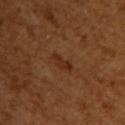Notes:
- tile lighting: cross-polarized
- anatomic site: the upper back
- diameter: about 3 mm
- image source: total-body-photography crop, ~15 mm field of view
- image-analysis metrics: an area of roughly 4 mm², a shape eccentricity near 0.9, and two-axis asymmetry of about 0.3; a border-irregularity index near 4/10, a within-lesion color-variation index near 1.5/10, and a peripheral color-asymmetry measure near 0.5; a detector confidence of about 100 out of 100 that the crop contains a lesion
- subject: female, in their mid-50s